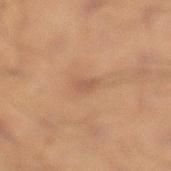Q: Who is the patient?
A: male, aged 38–42
Q: Where on the body is the lesion?
A: the left lower leg
Q: What is the imaging modality?
A: ~15 mm crop, total-body skin-cancer survey
Q: What did automated image analysis measure?
A: an average lesion color of about L≈49 a*≈19 b*≈28 (CIELAB), roughly 6 lightness units darker than nearby skin, and a normalized lesion–skin contrast near 5